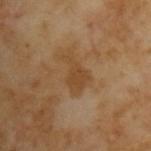Impression: Imaged during a routine full-body skin examination; the lesion was not biopsied and no histopathology is available. Context: A lesion tile, about 15 mm wide, cut from a 3D total-body photograph. A male patient about 65 years old.